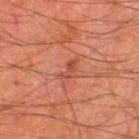Recorded during total-body skin imaging; not selected for excision or biopsy. The total-body-photography lesion software estimated an area of roughly 2.5 mm², a shape eccentricity near 0.9, and a shape-asymmetry score of about 0.45 (0 = symmetric). It also reported a mean CIELAB color near L≈44 a*≈28 b*≈30, a lesion–skin lightness drop of about 8, and a lesion-to-skin contrast of about 6 (normalized; higher = more distinct). The analysis additionally found a nevus-likeness score of about 0/100. A 15 mm close-up tile from a total-body photography series done for melanoma screening. On the left thigh. The patient is a male in their mid-60s.